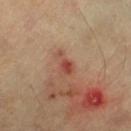{
  "lighting": "cross-polarized",
  "patient": {
    "age_approx": 60
  },
  "site": "right lower leg",
  "image": {
    "source": "total-body photography crop",
    "field_of_view_mm": 15
  },
  "lesion_size": {
    "long_diameter_mm_approx": 3.5
  }
}